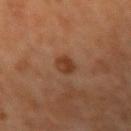No biopsy was performed on this lesion — it was imaged during a full skin examination and was not determined to be concerning. The subject is a female aged around 70. Captured under cross-polarized illumination. The lesion is located on the right forearm. A 15 mm close-up tile from a total-body photography series done for melanoma screening. Approximately 2.5 mm at its widest. Automated tile analysis of the lesion measured an automated nevus-likeness rating near 90 out of 100 and lesion-presence confidence of about 100/100.